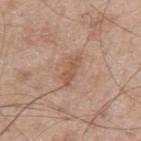| field | value |
|---|---|
| biopsy status | no biopsy performed (imaged during a skin exam) |
| tile lighting | white-light illumination |
| anatomic site | the arm |
| acquisition | ~15 mm tile from a whole-body skin photo |
| patient | male, approximately 65 years of age |
| size | ≈3.5 mm |
| image-analysis metrics | border irregularity of about 4.5 on a 0–10 scale, a color-variation rating of about 2/10, and peripheral color asymmetry of about 0.5 |